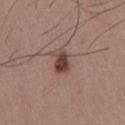Captured during whole-body skin photography for melanoma surveillance; the lesion was not biopsied. A 15 mm crop from a total-body photograph taken for skin-cancer surveillance. A male subject in their mid-50s. Located on the chest.